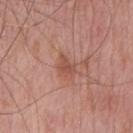Part of a total-body skin-imaging series; this lesion was reviewed on a skin check and was not flagged for biopsy. The patient is a male aged 73 to 77. This image is a 15 mm lesion crop taken from a total-body photograph. The lesion is located on the chest.